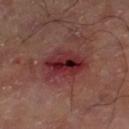The lesion was tiled from a total-body skin photograph and was not biopsied.